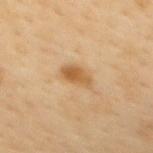A lesion tile, about 15 mm wide, cut from a 3D total-body photograph. Measured at roughly 3 mm in maximum diameter. This is a cross-polarized tile. The subject is a female roughly 50 years of age. The lesion is on the back.